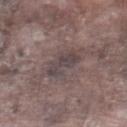| field | value |
|---|---|
| workup | no biopsy performed (imaged during a skin exam) |
| subject | male, approximately 75 years of age |
| automated lesion analysis | a lesion area of about 8 mm², an outline eccentricity of about 0.75 (0 = round, 1 = elongated), and a shape-asymmetry score of about 0.25 (0 = symmetric); an average lesion color of about L≈42 a*≈12 b*≈14 (CIELAB), about 7 CIELAB-L* units darker than the surrounding skin, and a lesion-to-skin contrast of about 6.5 (normalized; higher = more distinct); a border-irregularity index near 4/10, a within-lesion color-variation index near 3/10, and radial color variation of about 1 |
| body site | the right lower leg |
| lesion diameter | ≈4 mm |
| imaging modality | 15 mm crop, total-body photography |